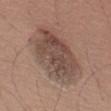follow-up=catalogued during a skin exam; not biopsied
imaging modality=~15 mm tile from a whole-body skin photo
location=the left thigh
subject=male, roughly 60 years of age
size=~8.5 mm (longest diameter)
illumination=white-light